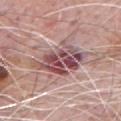{
  "biopsy_status": "not biopsied; imaged during a skin examination",
  "automated_metrics": {
    "cielab_L": 51,
    "cielab_a": 23,
    "cielab_b": 18,
    "vs_skin_darker_L": 15.0,
    "nevus_likeness_0_100": 0,
    "lesion_detection_confidence_0_100": 60
  },
  "lesion_size": {
    "long_diameter_mm_approx": 7.5
  },
  "site": "front of the torso",
  "lighting": "white-light",
  "patient": {
    "sex": "male",
    "age_approx": 80
  },
  "image": {
    "source": "total-body photography crop",
    "field_of_view_mm": 15
  }
}